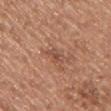  biopsy_status: not biopsied; imaged during a skin examination
  site: chest
  image:
    source: total-body photography crop
    field_of_view_mm: 15
  patient:
    sex: male
    age_approx: 75
  lighting: white-light